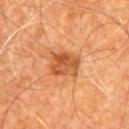Clinical impression:
The lesion was photographed on a routine skin check and not biopsied; there is no pathology result.
Acquisition and patient details:
The patient is a male aged around 60. A region of skin cropped from a whole-body photographic capture, roughly 15 mm wide. The lesion is on the chest. This is a cross-polarized tile. Approximately 3.5 mm at its widest.